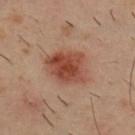Impression: This lesion was catalogued during total-body skin photography and was not selected for biopsy. Background: The tile uses cross-polarized illumination. Automated tile analysis of the lesion measured a lesion area of about 15 mm² and an outline eccentricity of about 0.6 (0 = round, 1 = elongated). The analysis additionally found a lesion color around L≈45 a*≈25 b*≈29 in CIELAB, a lesion–skin lightness drop of about 12, and a normalized border contrast of about 9. It also reported a border-irregularity index near 2/10 and internal color variation of about 5.5 on a 0–10 scale. From the upper back. A male subject aged around 40. Approximately 5 mm at its widest. A 15 mm crop from a total-body photograph taken for skin-cancer surveillance.